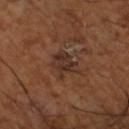Imaged during a routine full-body skin examination; the lesion was not biopsied and no histopathology is available. A region of skin cropped from a whole-body photographic capture, roughly 15 mm wide. On the left lower leg. A male subject, aged around 65.